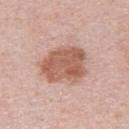Imaged during a routine full-body skin examination; the lesion was not biopsied and no histopathology is available.
About 6 mm across.
A 15 mm crop from a total-body photograph taken for skin-cancer surveillance.
A male subject, roughly 30 years of age.
Captured under white-light illumination.
An algorithmic analysis of the crop reported a footprint of about 19 mm², an outline eccentricity of about 0.7 (0 = round, 1 = elongated), and a shape-asymmetry score of about 0.2 (0 = symmetric). And it measured internal color variation of about 4 on a 0–10 scale.